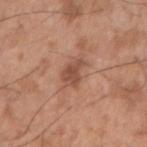workup: no biopsy performed (imaged during a skin exam)
acquisition: total-body-photography crop, ~15 mm field of view
lesion diameter: ≈3 mm
site: the arm
TBP lesion metrics: an area of roughly 5 mm², an outline eccentricity of about 0.7 (0 = round, 1 = elongated), and a shape-asymmetry score of about 0.45 (0 = symmetric); a mean CIELAB color near L≈49 a*≈23 b*≈29 and roughly 10 lightness units darker than nearby skin; an automated nevus-likeness rating near 5 out of 100 and lesion-presence confidence of about 100/100
tile lighting: white-light illumination
subject: male, aged approximately 50